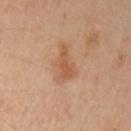This lesion was catalogued during total-body skin photography and was not selected for biopsy.
On the left upper arm.
This is a cross-polarized tile.
A region of skin cropped from a whole-body photographic capture, roughly 15 mm wide.
Automated tile analysis of the lesion measured a color-variation rating of about 2/10 and a peripheral color-asymmetry measure near 0.5. The software also gave a detector confidence of about 100 out of 100 that the crop contains a lesion.
The patient is a female in their 40s.
Approximately 4.5 mm at its widest.